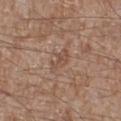The lesion was tiled from a total-body skin photograph and was not biopsied.
A male subject, about 65 years old.
A 15 mm close-up tile from a total-body photography series done for melanoma screening.
Automated image analysis of the tile measured an average lesion color of about L≈49 a*≈19 b*≈27 (CIELAB) and about 7 CIELAB-L* units darker than the surrounding skin. It also reported a border-irregularity index near 5/10.
The recorded lesion diameter is about 3 mm.
From the right lower leg.